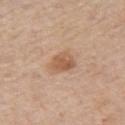The lesion was photographed on a routine skin check and not biopsied; there is no pathology result.
This is a white-light tile.
The subject is a male aged approximately 75.
A 15 mm close-up extracted from a 3D total-body photography capture.
On the left upper arm.
Measured at roughly 3 mm in maximum diameter.